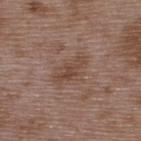* patient — male, about 50 years old
* image source — ~15 mm crop, total-body skin-cancer survey
* illumination — white-light
* location — the upper back
* TBP lesion metrics — a lesion area of about 9 mm² and two-axis asymmetry of about 0.25; a mean CIELAB color near L≈46 a*≈17 b*≈25, about 7 CIELAB-L* units darker than the surrounding skin, and a normalized border contrast of about 6
* lesion diameter — ~4.5 mm (longest diameter)